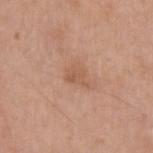No biopsy was performed on this lesion — it was imaged during a full skin examination and was not determined to be concerning.
A male subject, aged approximately 60.
Imaged with white-light lighting.
A 15 mm close-up tile from a total-body photography series done for melanoma screening.
On the left upper arm.